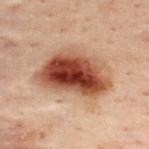follow-up = imaged on a skin check; not biopsied
body site = the upper back
image = ~15 mm crop, total-body skin-cancer survey
size = ~8 mm (longest diameter)
subject = male, roughly 50 years of age
tile lighting = cross-polarized